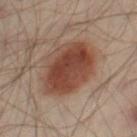This lesion was catalogued during total-body skin photography and was not selected for biopsy. Located on the left leg. Cropped from a total-body skin-imaging series; the visible field is about 15 mm. The patient is a male aged approximately 50. The total-body-photography lesion software estimated an area of roughly 28 mm², an eccentricity of roughly 0.7, and a symmetry-axis asymmetry near 0.1. The analysis additionally found a border-irregularity rating of about 1/10, internal color variation of about 5 on a 0–10 scale, and peripheral color asymmetry of about 1.5.